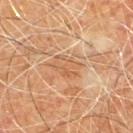Part of a total-body skin-imaging series; this lesion was reviewed on a skin check and was not flagged for biopsy. A 15 mm crop from a total-body photograph taken for skin-cancer surveillance. The lesion's longest dimension is about 3.5 mm. Imaged with cross-polarized lighting. An algorithmic analysis of the crop reported a lesion color around L≈54 a*≈20 b*≈34 in CIELAB, roughly 6 lightness units darker than nearby skin, and a normalized lesion–skin contrast near 5. It also reported a border-irregularity rating of about 4/10, a color-variation rating of about 3/10, and peripheral color asymmetry of about 1. A male subject aged around 60. The lesion is on the chest.